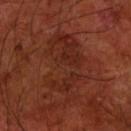Q: Is there a histopathology result?
A: imaged on a skin check; not biopsied
Q: Lesion size?
A: about 8.5 mm
Q: Who is the patient?
A: male, aged approximately 70
Q: What lighting was used for the tile?
A: cross-polarized
Q: What is the imaging modality?
A: 15 mm crop, total-body photography
Q: Lesion location?
A: the front of the torso